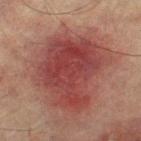workup=imaged on a skin check; not biopsied | body site=the left thigh | subject=male, in their mid-70s | lesion size=~8.5 mm (longest diameter) | acquisition=~15 mm tile from a whole-body skin photo | illumination=cross-polarized illumination.